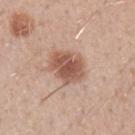<lesion>
<biopsy_status>not biopsied; imaged during a skin examination</biopsy_status>
<site>left forearm</site>
<patient>
  <sex>male</sex>
  <age_approx>30</age_approx>
</patient>
<lighting>white-light</lighting>
<lesion_size>
  <long_diameter_mm_approx>4.0</long_diameter_mm_approx>
</lesion_size>
<image>
  <source>total-body photography crop</source>
  <field_of_view_mm>15</field_of_view_mm>
</image>
</lesion>